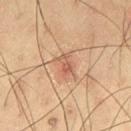No biopsy was performed on this lesion — it was imaged during a full skin examination and was not determined to be concerning. The tile uses cross-polarized illumination. The lesion is on the leg. A lesion tile, about 15 mm wide, cut from a 3D total-body photograph. The recorded lesion diameter is about 3 mm. The patient is a male roughly 35 years of age.